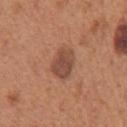  patient:
    sex: male
    age_approx: 65
  image:
    source: total-body photography crop
    field_of_view_mm: 15
  automated_metrics:
    vs_skin_darker_L: 10.0
    vs_skin_contrast_norm: 8.0
    border_irregularity_0_10: 2.0
    color_variation_0_10: 2.5
    peripheral_color_asymmetry: 1.0
  site: chest
  lighting: white-light
  lesion_size:
    long_diameter_mm_approx: 3.5